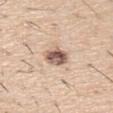Clinical impression:
Part of a total-body skin-imaging series; this lesion was reviewed on a skin check and was not flagged for biopsy.
Acquisition and patient details:
The recorded lesion diameter is about 3 mm. The lesion is located on the right upper arm. The total-body-photography lesion software estimated an area of roughly 6.5 mm², an outline eccentricity of about 0.45 (0 = round, 1 = elongated), and a symmetry-axis asymmetry near 0.2. A male subject about 60 years old. A 15 mm close-up tile from a total-body photography series done for melanoma screening. Imaged with white-light lighting.